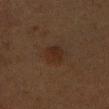No biopsy was performed on this lesion — it was imaged during a full skin examination and was not determined to be concerning. The lesion is located on the right upper arm. A 15 mm close-up tile from a total-body photography series done for melanoma screening. The patient is a female about 50 years old. This is a cross-polarized tile. An algorithmic analysis of the crop reported an average lesion color of about L≈21 a*≈14 b*≈21 (CIELAB), roughly 4 lightness units darker than nearby skin, and a normalized lesion–skin contrast near 6. It also reported a border-irregularity index near 1.5/10 and a within-lesion color-variation index near 2/10. About 3 mm across.